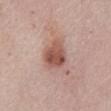This lesion was catalogued during total-body skin photography and was not selected for biopsy.
A 15 mm close-up tile from a total-body photography series done for melanoma screening.
The lesion is located on the front of the torso.
A male subject aged 63–67.